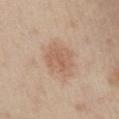Impression: The lesion was photographed on a routine skin check and not biopsied; there is no pathology result. Clinical summary: A male patient, in their 60s. Captured under white-light illumination. Cropped from a total-body skin-imaging series; the visible field is about 15 mm. From the chest. Approximately 4.5 mm at its widest. Automated tile analysis of the lesion measured a footprint of about 11 mm² and a shape eccentricity near 0.7. It also reported a border-irregularity index near 2.5/10 and peripheral color asymmetry of about 0.5.